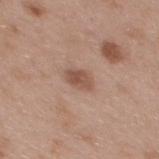| field | value |
|---|---|
| patient | female, aged approximately 40 |
| TBP lesion metrics | a footprint of about 4.5 mm², an eccentricity of roughly 0.75, and two-axis asymmetry of about 0.2; a within-lesion color-variation index near 3/10 and a peripheral color-asymmetry measure near 1 |
| site | the mid back |
| lesion size | about 3 mm |
| illumination | white-light |
| image | ~15 mm crop, total-body skin-cancer survey |